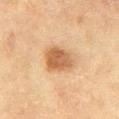Impression:
The lesion was photographed on a routine skin check and not biopsied; there is no pathology result.
Image and clinical context:
A female subject approximately 55 years of age. A 15 mm close-up extracted from a 3D total-body photography capture. The tile uses cross-polarized illumination. Measured at roughly 4 mm in maximum diameter. The lesion is on the right thigh.